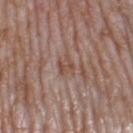Part of a total-body skin-imaging series; this lesion was reviewed on a skin check and was not flagged for biopsy.
A roughly 15 mm field-of-view crop from a total-body skin photograph.
This is a white-light tile.
The lesion is located on the left thigh.
A female patient, aged around 60.
Automated tile analysis of the lesion measured an area of roughly 4 mm², an eccentricity of roughly 0.5, and two-axis asymmetry of about 0.3. And it measured a detector confidence of about 65 out of 100 that the crop contains a lesion.